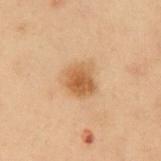Q: Was this lesion biopsied?
A: imaged on a skin check; not biopsied
Q: What kind of image is this?
A: ~15 mm crop, total-body skin-cancer survey
Q: How was the tile lit?
A: cross-polarized
Q: What is the lesion's diameter?
A: about 3.5 mm
Q: Lesion location?
A: the abdomen
Q: Automated lesion metrics?
A: an average lesion color of about L≈48 a*≈17 b*≈33 (CIELAB), a lesion–skin lightness drop of about 9, and a lesion-to-skin contrast of about 7.5 (normalized; higher = more distinct)
Q: Patient demographics?
A: male, roughly 55 years of age